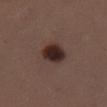Q: Was a biopsy performed?
A: imaged on a skin check; not biopsied
Q: Who is the patient?
A: female, about 40 years old
Q: Where on the body is the lesion?
A: the leg
Q: How was this image acquired?
A: 15 mm crop, total-body photography